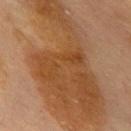workup — catalogued during a skin exam; not biopsied | image source — ~15 mm crop, total-body skin-cancer survey | diameter — about 19 mm | subject — female, roughly 60 years of age | site — the chest.